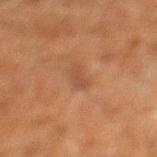{"biopsy_status": "not biopsied; imaged during a skin examination", "lighting": "cross-polarized", "site": "leg", "lesion_size": {"long_diameter_mm_approx": 3.0}, "patient": {"sex": "male", "age_approx": 60}, "image": {"source": "total-body photography crop", "field_of_view_mm": 15}}